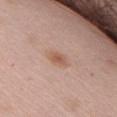follow-up = no biopsy performed (imaged during a skin exam) | subject = male, aged 63–67 | tile lighting = white-light illumination | lesion diameter = ≈2.5 mm | anatomic site = the chest | automated metrics = a lesion color around L≈57 a*≈21 b*≈29 in CIELAB, roughly 9 lightness units darker than nearby skin, and a normalized border contrast of about 6.5; a within-lesion color-variation index near 2/10 and a peripheral color-asymmetry measure near 0.5; a classifier nevus-likeness of about 80/100 and lesion-presence confidence of about 100/100 | image source = 15 mm crop, total-body photography.